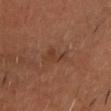<case>
<biopsy_status>not biopsied; imaged during a skin examination</biopsy_status>
<patient>
  <sex>male</sex>
  <age_approx>60</age_approx>
</patient>
<site>head or neck</site>
<image>
  <source>total-body photography crop</source>
  <field_of_view_mm>15</field_of_view_mm>
</image>
<lesion_size>
  <long_diameter_mm_approx>2.5</long_diameter_mm_approx>
</lesion_size>
</case>